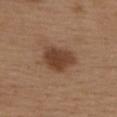Impression: The lesion was photographed on a routine skin check and not biopsied; there is no pathology result. Clinical summary: Automated image analysis of the tile measured about 11 CIELAB-L* units darker than the surrounding skin and a normalized border contrast of about 9. It also reported internal color variation of about 3 on a 0–10 scale and peripheral color asymmetry of about 1. The analysis additionally found an automated nevus-likeness rating near 85 out of 100 and a lesion-detection confidence of about 100/100. This image is a 15 mm lesion crop taken from a total-body photograph. A female subject aged approximately 40. The lesion is located on the back.